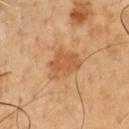Part of a total-body skin-imaging series; this lesion was reviewed on a skin check and was not flagged for biopsy. The lesion is on the front of the torso. The patient is a male about 50 years old. Cropped from a whole-body photographic skin survey; the tile spans about 15 mm.